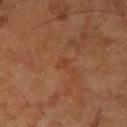Assessment: The lesion was tiled from a total-body skin photograph and was not biopsied. Context: This image is a 15 mm lesion crop taken from a total-body photograph. An algorithmic analysis of the crop reported border irregularity of about 2.5 on a 0–10 scale and peripheral color asymmetry of about 0. The lesion is located on the left forearm. Imaged with cross-polarized lighting. The patient is a female aged 68–72.